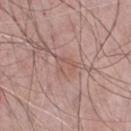Findings:
- biopsy status: total-body-photography surveillance lesion; no biopsy
- subject: male, in their mid- to late 60s
- image-analysis metrics: a footprint of about 5 mm², an outline eccentricity of about 0.5 (0 = round, 1 = elongated), and two-axis asymmetry of about 0.35; lesion-presence confidence of about 65/100
- diameter: about 3 mm
- location: the chest
- image: ~15 mm crop, total-body skin-cancer survey
- lighting: white-light illumination